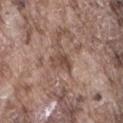This lesion was catalogued during total-body skin photography and was not selected for biopsy. The tile uses white-light illumination. The lesion is located on the lower back. A roughly 15 mm field-of-view crop from a total-body skin photograph. A male patient aged 73 to 77. Measured at roughly 3 mm in maximum diameter.